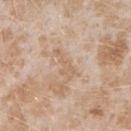No biopsy was performed on this lesion — it was imaged during a full skin examination and was not determined to be concerning. A female subject aged around 25. The recorded lesion diameter is about 4 mm. On the right forearm. A region of skin cropped from a whole-body photographic capture, roughly 15 mm wide.